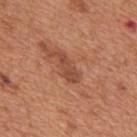Case summary:
– notes: no biopsy performed (imaged during a skin exam)
– image: 15 mm crop, total-body photography
– patient: male, aged approximately 65
– illumination: white-light illumination
– body site: the mid back
– lesion size: about 3.5 mm